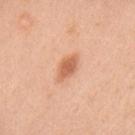Assessment: The lesion was photographed on a routine skin check and not biopsied; there is no pathology result. Image and clinical context: About 3.5 mm across. The lesion is located on the upper back. Captured under white-light illumination. This image is a 15 mm lesion crop taken from a total-body photograph. The subject is a female aged around 40.